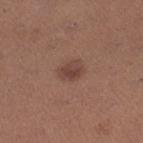Recorded during total-body skin imaging; not selected for excision or biopsy. From the right lower leg. A roughly 15 mm field-of-view crop from a total-body skin photograph. A female patient about 30 years old. Measured at roughly 2.5 mm in maximum diameter.